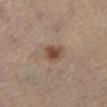Case summary:
• biopsy status: catalogued during a skin exam; not biopsied
• imaging modality: total-body-photography crop, ~15 mm field of view
• subject: male, roughly 50 years of age
• TBP lesion metrics: a lesion color around L≈46 a*≈16 b*≈26 in CIELAB, roughly 10 lightness units darker than nearby skin, and a normalized lesion–skin contrast near 8.5; border irregularity of about 2 on a 0–10 scale, a within-lesion color-variation index near 2.5/10, and a peripheral color-asymmetry measure near 0.5
• lesion diameter: about 3 mm
• body site: the left leg
• tile lighting: cross-polarized illumination